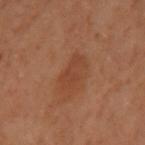biopsy_status: not biopsied; imaged during a skin examination
site: left arm
lighting: cross-polarized
automated_metrics:
  border_irregularity_0_10: 3.0
  color_variation_0_10: 1.5
  peripheral_color_asymmetry: 0.5
  lesion_detection_confidence_0_100: 100
lesion_size:
  long_diameter_mm_approx: 3.5
image:
  source: total-body photography crop
  field_of_view_mm: 15
patient:
  sex: female
  age_approx: 60